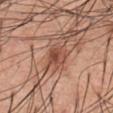workup: no biopsy performed (imaged during a skin exam) | size: ~2.5 mm (longest diameter) | site: the abdomen | subject: male, roughly 55 years of age | tile lighting: white-light illumination | acquisition: ~15 mm tile from a whole-body skin photo.